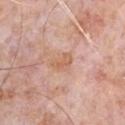Impression: Recorded during total-body skin imaging; not selected for excision or biopsy. Clinical summary: An algorithmic analysis of the crop reported a footprint of about 3.5 mm² and a symmetry-axis asymmetry near 0.25. And it measured a nevus-likeness score of about 0/100 and a lesion-detection confidence of about 100/100. A region of skin cropped from a whole-body photographic capture, roughly 15 mm wide. Measured at roughly 3 mm in maximum diameter. The patient is a male roughly 80 years of age. The lesion is located on the chest. Imaged with white-light lighting.